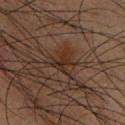Captured during whole-body skin photography for melanoma surveillance; the lesion was not biopsied. A region of skin cropped from a whole-body photographic capture, roughly 15 mm wide. A male patient aged 33–37. From the chest. The recorded lesion diameter is about 3.5 mm. The total-body-photography lesion software estimated a footprint of about 5 mm² and a symmetry-axis asymmetry near 0.5. This is a cross-polarized tile.